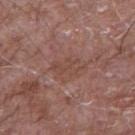{
  "biopsy_status": "not biopsied; imaged during a skin examination"
}